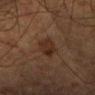Impression:
This lesion was catalogued during total-body skin photography and was not selected for biopsy.
Image and clinical context:
A male patient, approximately 45 years of age. This is a cross-polarized tile. Cropped from a whole-body photographic skin survey; the tile spans about 15 mm. Measured at roughly 4 mm in maximum diameter. Located on the head or neck.